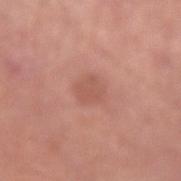Imaged during a routine full-body skin examination; the lesion was not biopsied and no histopathology is available.
The lesion is on the arm.
The tile uses white-light illumination.
The recorded lesion diameter is about 3 mm.
A male patient, aged 78–82.
Cropped from a whole-body photographic skin survey; the tile spans about 15 mm.